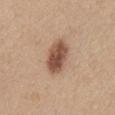notes: catalogued during a skin exam; not biopsied | tile lighting: white-light illumination | patient: male, aged approximately 30 | site: the abdomen | lesion size: about 5 mm | image source: ~15 mm tile from a whole-body skin photo.